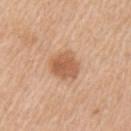follow-up: catalogued during a skin exam; not biopsied
lesion size: ≈3.5 mm
location: the arm
patient: male, about 60 years old
acquisition: 15 mm crop, total-body photography